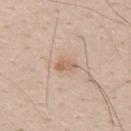Notes:
* follow-up: no biopsy performed (imaged during a skin exam)
* tile lighting: white-light
* size: ~2.5 mm (longest diameter)
* body site: the back
* TBP lesion metrics: a mean CIELAB color near L≈62 a*≈19 b*≈30, roughly 9 lightness units darker than nearby skin, and a normalized border contrast of about 6; border irregularity of about 2.5 on a 0–10 scale, a color-variation rating of about 3/10, and a peripheral color-asymmetry measure near 1
* image source: 15 mm crop, total-body photography
* patient: male, roughly 35 years of age